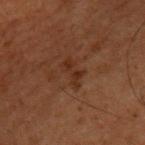Acquisition and patient details: A male patient aged 58 to 62. Imaged with cross-polarized lighting. Located on the upper back. This image is a 15 mm lesion crop taken from a total-body photograph. The lesion's longest dimension is about 3.5 mm.